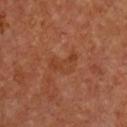The total-body-photography lesion software estimated two-axis asymmetry of about 0.5. And it measured a mean CIELAB color near L≈40 a*≈25 b*≈33 and a normalized border contrast of about 5. The analysis additionally found a border-irregularity rating of about 5.5/10, internal color variation of about 2 on a 0–10 scale, and peripheral color asymmetry of about 0.5. The analysis additionally found a nevus-likeness score of about 0/100 and a lesion-detection confidence of about 100/100. Imaged with cross-polarized lighting. Cropped from a whole-body photographic skin survey; the tile spans about 15 mm. A female patient, aged approximately 65. The lesion is located on the chest.